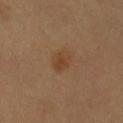| field | value |
|---|---|
| biopsy status | no biopsy performed (imaged during a skin exam) |
| anatomic site | the left forearm |
| diameter | ~2.5 mm (longest diameter) |
| subject | female, in their 60s |
| image | ~15 mm tile from a whole-body skin photo |
| lighting | cross-polarized |